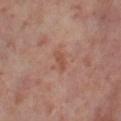Impression:
The lesion was photographed on a routine skin check and not biopsied; there is no pathology result.
Clinical summary:
The lesion is located on the leg. Cropped from a whole-body photographic skin survey; the tile spans about 15 mm. The patient is a female aged 53–57. An algorithmic analysis of the crop reported a footprint of about 2 mm², an outline eccentricity of about 0.9 (0 = round, 1 = elongated), and a symmetry-axis asymmetry near 0.45. The software also gave border irregularity of about 4.5 on a 0–10 scale, internal color variation of about 0 on a 0–10 scale, and a peripheral color-asymmetry measure near 0.